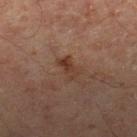  biopsy_status: not biopsied; imaged during a skin examination
  patient:
    sex: male
    age_approx: 65
  lighting: cross-polarized
  lesion_size:
    long_diameter_mm_approx: 3.0
  site: leg
  image:
    source: total-body photography crop
    field_of_view_mm: 15
  automated_metrics:
    shape_asymmetry: 0.2
    vs_skin_darker_L: 6.0